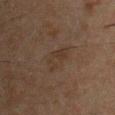Recorded during total-body skin imaging; not selected for excision or biopsy. A roughly 15 mm field-of-view crop from a total-body skin photograph. The lesion is located on the chest. A male patient, aged 48 to 52. The lesion's longest dimension is about 3.5 mm.